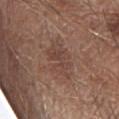follow-up=catalogued during a skin exam; not biopsied
body site=the right forearm
image-analysis metrics=a footprint of about 9 mm², a shape eccentricity near 0.75, and a symmetry-axis asymmetry near 0.3
diameter=~4.5 mm (longest diameter)
image source=total-body-photography crop, ~15 mm field of view
subject=male, roughly 55 years of age